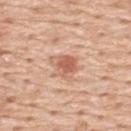No biopsy was performed on this lesion — it was imaged during a full skin examination and was not determined to be concerning. Cropped from a total-body skin-imaging series; the visible field is about 15 mm. A male patient approximately 60 years of age. Located on the back.